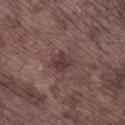Q: Was a biopsy performed?
A: catalogued during a skin exam; not biopsied
Q: Patient demographics?
A: male, in their mid- to late 70s
Q: How was this image acquired?
A: ~15 mm tile from a whole-body skin photo
Q: What is the lesion's diameter?
A: about 2.5 mm
Q: What did automated image analysis measure?
A: a border-irregularity rating of about 2/10, a within-lesion color-variation index near 3/10, and radial color variation of about 1; a nevus-likeness score of about 5/100
Q: What is the anatomic site?
A: the right lower leg